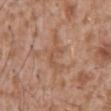Q: Is there a histopathology result?
A: imaged on a skin check; not biopsied
Q: Lesion size?
A: about 5 mm
Q: What kind of image is this?
A: ~15 mm crop, total-body skin-cancer survey
Q: Lesion location?
A: the abdomen
Q: How was the tile lit?
A: white-light illumination
Q: What are the patient's age and sex?
A: male, aged 43–47